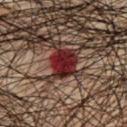Assessment:
Part of a total-body skin-imaging series; this lesion was reviewed on a skin check and was not flagged for biopsy.
Background:
From the chest. A region of skin cropped from a whole-body photographic capture, roughly 15 mm wide. Automated image analysis of the tile measured a lesion area of about 8 mm² and an outline eccentricity of about 0.55 (0 = round, 1 = elongated). And it measured a lesion color around L≈25 a*≈30 b*≈21 in CIELAB, about 15 CIELAB-L* units darker than the surrounding skin, and a lesion-to-skin contrast of about 15 (normalized; higher = more distinct). It also reported a classifier nevus-likeness of about 0/100. A male subject in their mid- to late 40s. Captured under cross-polarized illumination. The lesion's longest dimension is about 3.5 mm.